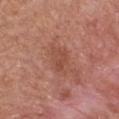{
  "biopsy_status": "not biopsied; imaged during a skin examination",
  "lesion_size": {
    "long_diameter_mm_approx": 3.5
  },
  "lighting": "white-light",
  "site": "chest",
  "image": {
    "source": "total-body photography crop",
    "field_of_view_mm": 15
  },
  "automated_metrics": {
    "cielab_L": 48,
    "cielab_a": 25,
    "cielab_b": 29,
    "vs_skin_darker_L": 7.0,
    "vs_skin_contrast_norm": 5.5,
    "border_irregularity_0_10": 4.0,
    "color_variation_0_10": 2.0
  },
  "patient": {
    "sex": "female",
    "age_approx": 40
  }
}